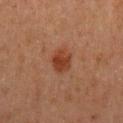Impression: The lesion was tiled from a total-body skin photograph and was not biopsied. Context: The subject is a male aged approximately 65. Located on the mid back. A 15 mm crop from a total-body photograph taken for skin-cancer surveillance. Longest diameter approximately 3 mm. The tile uses cross-polarized illumination.